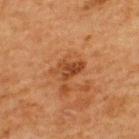Q: Was a biopsy performed?
A: catalogued during a skin exam; not biopsied